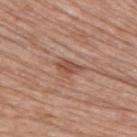Findings:
– workup: no biopsy performed (imaged during a skin exam)
– anatomic site: the upper back
– image: total-body-photography crop, ~15 mm field of view
– subject: male, aged approximately 85 or older
– lesion size: about 3.5 mm